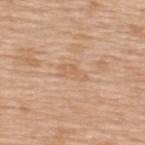Captured during whole-body skin photography for melanoma surveillance; the lesion was not biopsied. A 15 mm crop from a total-body photograph taken for skin-cancer surveillance. The subject is a female roughly 45 years of age. Approximately 3.5 mm at its widest. On the upper back. Automated image analysis of the tile measured a lesion area of about 4 mm² and a shape eccentricity near 0.9. Imaged with white-light lighting.